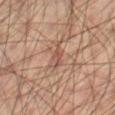The lesion was photographed on a routine skin check and not biopsied; there is no pathology result.
The subject is a male about 60 years old.
On the leg.
A 15 mm close-up tile from a total-body photography series done for melanoma screening.